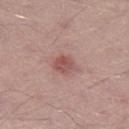Q: Is there a histopathology result?
A: total-body-photography surveillance lesion; no biopsy
Q: How was the tile lit?
A: white-light
Q: How was this image acquired?
A: ~15 mm tile from a whole-body skin photo
Q: Lesion location?
A: the right thigh
Q: What are the patient's age and sex?
A: male, aged around 40